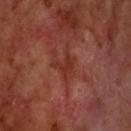notes: total-body-photography surveillance lesion; no biopsy
image source: ~15 mm tile from a whole-body skin photo
subject: male, approximately 70 years of age
anatomic site: the head or neck
lighting: cross-polarized